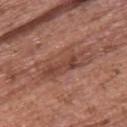The lesion was tiled from a total-body skin photograph and was not biopsied. Located on the upper back. A 15 mm close-up extracted from a 3D total-body photography capture. A female subject, aged approximately 65. Imaged with white-light lighting. Automated image analysis of the tile measured about 8 CIELAB-L* units darker than the surrounding skin and a lesion-to-skin contrast of about 6 (normalized; higher = more distinct). The analysis additionally found an automated nevus-likeness rating near 5 out of 100 and a lesion-detection confidence of about 65/100. Measured at roughly 7.5 mm in maximum diameter.